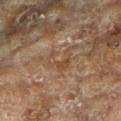biopsy status: catalogued during a skin exam; not biopsied | lesion diameter: ≈3 mm | anatomic site: the left arm | tile lighting: cross-polarized illumination | image source: total-body-photography crop, ~15 mm field of view | subject: female, aged 78–82 | TBP lesion metrics: a footprint of about 5 mm², an outline eccentricity of about 0.55 (0 = round, 1 = elongated), and two-axis asymmetry of about 0.8.